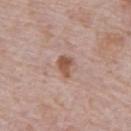Recorded during total-body skin imaging; not selected for excision or biopsy.
Captured under white-light illumination.
Approximately 2.5 mm at its widest.
Cropped from a total-body skin-imaging series; the visible field is about 15 mm.
Automated image analysis of the tile measured a shape-asymmetry score of about 0.35 (0 = symmetric). It also reported roughly 12 lightness units darker than nearby skin and a lesion-to-skin contrast of about 9 (normalized; higher = more distinct). The software also gave a nevus-likeness score of about 90/100 and a detector confidence of about 100 out of 100 that the crop contains a lesion.
The lesion is on the abdomen.
A male subject in their 70s.